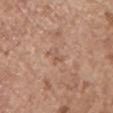| field | value |
|---|---|
| notes | no biopsy performed (imaged during a skin exam) |
| lighting | white-light |
| location | the left upper arm |
| imaging modality | ~15 mm tile from a whole-body skin photo |
| patient | female, aged around 65 |